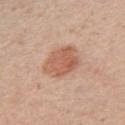The lesion was photographed on a routine skin check and not biopsied; there is no pathology result.